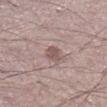biopsy status = no biopsy performed (imaged during a skin exam); diameter = ~2.5 mm (longest diameter); lighting = white-light; body site = the right lower leg; image source = total-body-photography crop, ~15 mm field of view; patient = male, roughly 60 years of age.